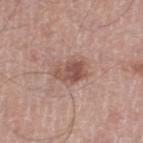Imaged during a routine full-body skin examination; the lesion was not biopsied and no histopathology is available.